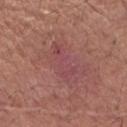Impression:
The lesion was tiled from a total-body skin photograph and was not biopsied.